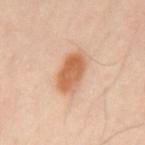Impression: The lesion was tiled from a total-body skin photograph and was not biopsied.